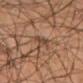No biopsy was performed on this lesion — it was imaged during a full skin examination and was not determined to be concerning.
A close-up tile cropped from a whole-body skin photograph, about 15 mm across.
A male patient, aged around 55.
This is a cross-polarized tile.
Measured at roughly 3 mm in maximum diameter.
On the leg.